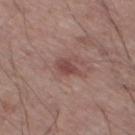The lesion was photographed on a routine skin check and not biopsied; there is no pathology result. A 15 mm close-up extracted from a 3D total-body photography capture. From the left lower leg. Imaged with white-light lighting. Measured at roughly 2.5 mm in maximum diameter. The patient is a male aged around 55. The total-body-photography lesion software estimated a lesion area of about 4 mm², an eccentricity of roughly 0.7, and a shape-asymmetry score of about 0.25 (0 = symmetric).